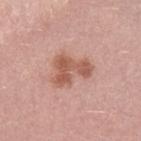{"biopsy_status": "not biopsied; imaged during a skin examination", "lighting": "white-light", "patient": {"sex": "male", "age_approx": 30}, "image": {"source": "total-body photography crop", "field_of_view_mm": 15}, "automated_metrics": {"area_mm2_approx": 11.0, "eccentricity": 0.7}}